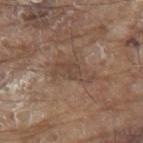<tbp_lesion>
<biopsy_status>not biopsied; imaged during a skin examination</biopsy_status>
<patient>
  <sex>male</sex>
  <age_approx>80</age_approx>
</patient>
<site>back</site>
<image>
  <source>total-body photography crop</source>
  <field_of_view_mm>15</field_of_view_mm>
</image>
</tbp_lesion>